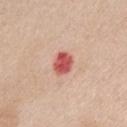Q: Was this lesion biopsied?
A: imaged on a skin check; not biopsied
Q: What are the patient's age and sex?
A: female, in their mid-60s
Q: What is the imaging modality?
A: total-body-photography crop, ~15 mm field of view
Q: What is the anatomic site?
A: the chest
Q: What is the lesion's diameter?
A: ~3 mm (longest diameter)